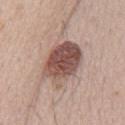Cropped from a whole-body photographic skin survey; the tile spans about 15 mm. A male patient, aged around 65. On the front of the torso.